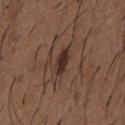Impression:
Imaged during a routine full-body skin examination; the lesion was not biopsied and no histopathology is available.
Acquisition and patient details:
A male subject, roughly 50 years of age. A 15 mm crop from a total-body photograph taken for skin-cancer surveillance. The lesion is on the chest.